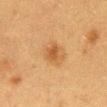Imaged during a routine full-body skin examination; the lesion was not biopsied and no histopathology is available.
The lesion is on the chest.
Longest diameter approximately 3 mm.
A lesion tile, about 15 mm wide, cut from a 3D total-body photograph.
A female subject, about 40 years old.